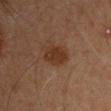Clinical summary:
The tile uses cross-polarized illumination. A lesion tile, about 15 mm wide, cut from a 3D total-body photograph. A male patient aged 43–47. The lesion is located on the left upper arm. Measured at roughly 3.5 mm in maximum diameter.